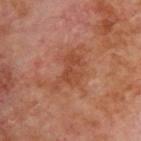Case summary:
- biopsy status · catalogued during a skin exam; not biopsied
- location · the upper back
- patient · male, in their 70s
- acquisition · total-body-photography crop, ~15 mm field of view
- automated lesion analysis · border irregularity of about 5.5 on a 0–10 scale, internal color variation of about 3 on a 0–10 scale, and a peripheral color-asymmetry measure near 1; a classifier nevus-likeness of about 0/100 and a lesion-detection confidence of about 100/100